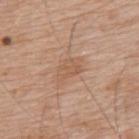Impression:
No biopsy was performed on this lesion — it was imaged during a full skin examination and was not determined to be concerning.
Context:
The lesion-visualizer software estimated an area of roughly 5.5 mm², an outline eccentricity of about 0.8 (0 = round, 1 = elongated), and a symmetry-axis asymmetry near 0.3. The analysis additionally found an average lesion color of about L≈57 a*≈20 b*≈32 (CIELAB) and a lesion-to-skin contrast of about 5 (normalized; higher = more distinct). And it measured a border-irregularity rating of about 3/10 and radial color variation of about 0.5. And it measured a classifier nevus-likeness of about 0/100 and lesion-presence confidence of about 100/100. The subject is a male aged 78–82. The tile uses white-light illumination. Cropped from a whole-body photographic skin survey; the tile spans about 15 mm. On the upper back.